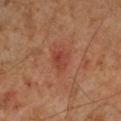patient = male, approximately 60 years of age | anatomic site = the left lower leg | image source = ~15 mm tile from a whole-body skin photo | diameter = about 2.5 mm | TBP lesion metrics = a border-irregularity index near 2/10 | illumination = cross-polarized.